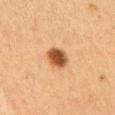notes: total-body-photography surveillance lesion; no biopsy | site: the right upper arm | subject: female, in their 40s | lesion diameter: ≈3.5 mm | image-analysis metrics: a footprint of about 6 mm², an outline eccentricity of about 0.75 (0 = round, 1 = elongated), and two-axis asymmetry of about 0.15; a lesion–skin lightness drop of about 17 and a lesion-to-skin contrast of about 12 (normalized; higher = more distinct); a peripheral color-asymmetry measure near 1; a lesion-detection confidence of about 100/100 | illumination: cross-polarized illumination | image source: 15 mm crop, total-body photography.